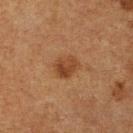* notes · no biopsy performed (imaged during a skin exam)
* subject · male, aged 73 to 77
* body site · the right lower leg
* image · 15 mm crop, total-body photography
* tile lighting · cross-polarized
* automated metrics · an eccentricity of roughly 0.55 and two-axis asymmetry of about 0.15; a lesion color around L≈34 a*≈19 b*≈29 in CIELAB, a lesion–skin lightness drop of about 8, and a normalized border contrast of about 7.5; a color-variation rating of about 4/10
* lesion diameter · about 3 mm